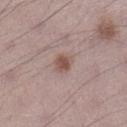{"site": "left lower leg", "lesion_size": {"long_diameter_mm_approx": 2.5}, "patient": {"sex": "male", "age_approx": 70}, "lighting": "white-light", "image": {"source": "total-body photography crop", "field_of_view_mm": 15}}